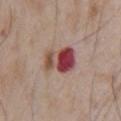{
  "biopsy_status": "not biopsied; imaged during a skin examination",
  "image": {
    "source": "total-body photography crop",
    "field_of_view_mm": 15
  },
  "automated_metrics": {
    "area_mm2_approx": 10.0,
    "eccentricity": 0.85,
    "border_irregularity_0_10": 3.0,
    "color_variation_0_10": 10.0,
    "peripheral_color_asymmetry": 7.0,
    "lesion_detection_confidence_0_100": 100
  },
  "patient": {
    "sex": "male",
    "age_approx": 65
  },
  "site": "chest",
  "lesion_size": {
    "long_diameter_mm_approx": 5.0
  },
  "lighting": "white-light"
}